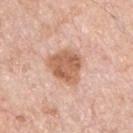| feature | finding |
|---|---|
| follow-up | no biopsy performed (imaged during a skin exam) |
| subject | male, aged approximately 70 |
| acquisition | total-body-photography crop, ~15 mm field of view |
| body site | the chest |